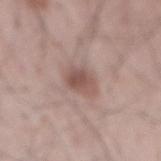workup = imaged on a skin check; not biopsied | imaging modality = ~15 mm tile from a whole-body skin photo | lighting = white-light illumination | subject = male, aged approximately 65 | body site = the mid back | size = ~3.5 mm (longest diameter) | image-analysis metrics = a lesion area of about 7 mm² and an eccentricity of roughly 0.7; a lesion–skin lightness drop of about 10 and a normalized lesion–skin contrast near 7.5.